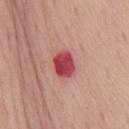<tbp_lesion>
<biopsy_status>not biopsied; imaged during a skin examination</biopsy_status>
<image>
  <source>total-body photography crop</source>
  <field_of_view_mm>15</field_of_view_mm>
</image>
<lighting>white-light</lighting>
<site>back</site>
<patient>
  <sex>female</sex>
  <age_approx>65</age_approx>
</patient>
</tbp_lesion>